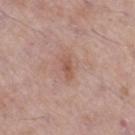Q: Is there a histopathology result?
A: imaged on a skin check; not biopsied
Q: How large is the lesion?
A: ~2.5 mm (longest diameter)
Q: What did automated image analysis measure?
A: a lesion area of about 3 mm², an outline eccentricity of about 0.9 (0 = round, 1 = elongated), and a symmetry-axis asymmetry near 0.35; a mean CIELAB color near L≈54 a*≈22 b*≈28, about 8 CIELAB-L* units darker than the surrounding skin, and a normalized lesion–skin contrast near 6.5; a nevus-likeness score of about 0/100 and lesion-presence confidence of about 100/100
Q: What are the patient's age and sex?
A: male, in their mid- to late 50s
Q: What lighting was used for the tile?
A: white-light
Q: What kind of image is this?
A: 15 mm crop, total-body photography
Q: Lesion location?
A: the right thigh